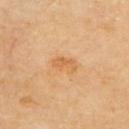Case summary:
• follow-up — imaged on a skin check; not biopsied
• diameter — ~3.5 mm (longest diameter)
• tile lighting — cross-polarized illumination
• site — the upper back
• imaging modality — ~15 mm crop, total-body skin-cancer survey
• subject — female, aged approximately 70
• automated metrics — an area of roughly 4.5 mm² and two-axis asymmetry of about 0.35; a mean CIELAB color near L≈65 a*≈23 b*≈44, roughly 8 lightness units darker than nearby skin, and a lesion-to-skin contrast of about 5.5 (normalized; higher = more distinct)